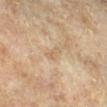Case summary:
- biopsy status: no biopsy performed (imaged during a skin exam)
- acquisition: 15 mm crop, total-body photography
- anatomic site: the right lower leg
- automated lesion analysis: an area of roughly 2.5 mm², an outline eccentricity of about 0.9 (0 = round, 1 = elongated), and a shape-asymmetry score of about 0.4 (0 = symmetric); border irregularity of about 4 on a 0–10 scale
- patient: male, aged 83 or older
- illumination: cross-polarized illumination